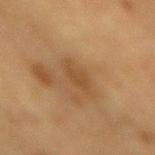biopsy_status: not biopsied; imaged during a skin examination
site: mid back
lesion_size:
  long_diameter_mm_approx: 3.5
lighting: cross-polarized
patient:
  sex: male
  age_approx: 85+
image:
  source: total-body photography crop
  field_of_view_mm: 15